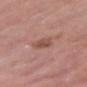Q: Was this lesion biopsied?
A: imaged on a skin check; not biopsied
Q: What is the imaging modality?
A: total-body-photography crop, ~15 mm field of view
Q: Who is the patient?
A: male, aged around 70
Q: How was the tile lit?
A: white-light illumination
Q: Lesion size?
A: ≈3.5 mm
Q: What is the anatomic site?
A: the head or neck